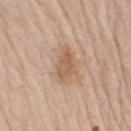{
  "biopsy_status": "not biopsied; imaged during a skin examination",
  "site": "arm",
  "automated_metrics": {
    "area_mm2_approx": 7.0,
    "eccentricity": 0.75,
    "shape_asymmetry": 0.25,
    "cielab_L": 59,
    "cielab_a": 18,
    "cielab_b": 32,
    "vs_skin_darker_L": 9.0,
    "vs_skin_contrast_norm": 6.5
  },
  "lighting": "white-light",
  "lesion_size": {
    "long_diameter_mm_approx": 3.5
  },
  "image": {
    "source": "total-body photography crop",
    "field_of_view_mm": 15
  },
  "patient": {
    "sex": "male",
    "age_approx": 75
  }
}